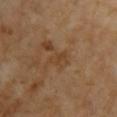Findings:
• biopsy status: catalogued during a skin exam; not biopsied
• anatomic site: the chest
• automated metrics: a footprint of about 3 mm², an outline eccentricity of about 0.85 (0 = round, 1 = elongated), and a shape-asymmetry score of about 0.3 (0 = symmetric); a border-irregularity index near 3.5/10 and a within-lesion color-variation index near 1.5/10; an automated nevus-likeness rating near 0 out of 100 and a detector confidence of about 100 out of 100 that the crop contains a lesion
• size: ~3 mm (longest diameter)
• illumination: cross-polarized illumination
• patient: female, aged around 70
• image: 15 mm crop, total-body photography